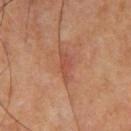Background: A male patient aged 63–67. Located on the chest. This image is a 15 mm lesion crop taken from a total-body photograph.